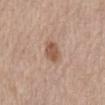imaging modality: total-body-photography crop, ~15 mm field of view | site: the abdomen | patient: female, aged 63–67.